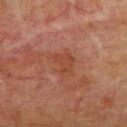Case summary:
* notes · imaged on a skin check; not biopsied
* TBP lesion metrics · a footprint of about 6.5 mm² and a shape eccentricity near 0.7; a within-lesion color-variation index near 2.5/10; an automated nevus-likeness rating near 0 out of 100 and a detector confidence of about 100 out of 100 that the crop contains a lesion
* image · total-body-photography crop, ~15 mm field of view
* location · the back
* size · ≈3.5 mm
* patient · male, approximately 60 years of age